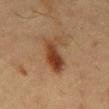| feature | finding |
|---|---|
| notes | catalogued during a skin exam; not biopsied |
| image | ~15 mm crop, total-body skin-cancer survey |
| illumination | cross-polarized |
| automated lesion analysis | an area of roughly 10 mm² and a shape eccentricity near 0.85; a lesion color around L≈32 a*≈17 b*≈27 in CIELAB, a lesion–skin lightness drop of about 10, and a lesion-to-skin contrast of about 9.5 (normalized; higher = more distinct) |
| body site | the back |
| subject | male, aged 58–62 |